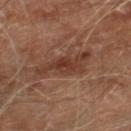Q: Was this lesion biopsied?
A: total-body-photography surveillance lesion; no biopsy
Q: Patient demographics?
A: male, approximately 70 years of age
Q: Illumination type?
A: cross-polarized
Q: How was this image acquired?
A: ~15 mm tile from a whole-body skin photo
Q: What is the anatomic site?
A: the right lower leg